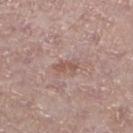biopsy_status: not biopsied; imaged during a skin examination
image:
  source: total-body photography crop
  field_of_view_mm: 15
site: leg
patient:
  sex: male
  age_approx: 65